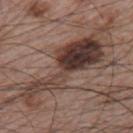{"biopsy_status": "not biopsied; imaged during a skin examination", "site": "left upper arm", "patient": {"sex": "male", "age_approx": 65}, "lighting": "white-light", "image": {"source": "total-body photography crop", "field_of_view_mm": 15}, "lesion_size": {"long_diameter_mm_approx": 11.0}, "automated_metrics": {"shape_asymmetry": 0.6, "cielab_L": 38, "cielab_a": 17, "cielab_b": 21, "vs_skin_darker_L": 14.0, "border_irregularity_0_10": 9.5, "color_variation_0_10": 9.0, "peripheral_color_asymmetry": 3.0, "nevus_likeness_0_100": 0, "lesion_detection_confidence_0_100": 95}}